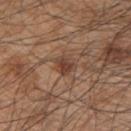Clinical impression:
Recorded during total-body skin imaging; not selected for excision or biopsy.
Background:
The lesion's longest dimension is about 3 mm. A male subject, aged approximately 45. From the back. A 15 mm close-up extracted from a 3D total-body photography capture. Captured under white-light illumination.